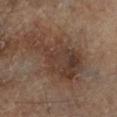Imaged during a routine full-body skin examination; the lesion was not biopsied and no histopathology is available. Measured at roughly 8.5 mm in maximum diameter. A 15 mm crop from a total-body photograph taken for skin-cancer surveillance. From the left lower leg. Automated tile analysis of the lesion measured an area of roughly 24 mm², a shape eccentricity near 0.85, and a symmetry-axis asymmetry near 0.5. The analysis additionally found an average lesion color of about L≈35 a*≈16 b*≈23 (CIELAB) and a lesion–skin lightness drop of about 8. This is a cross-polarized tile. A male subject aged around 65.